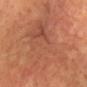The lesion was photographed on a routine skin check and not biopsied; there is no pathology result. A female subject, in their 70s. Cropped from a total-body skin-imaging series; the visible field is about 15 mm. The lesion is on the head or neck.